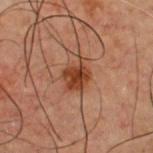The lesion was tiled from a total-body skin photograph and was not biopsied. On the chest. A close-up tile cropped from a whole-body skin photograph, about 15 mm across. Measured at roughly 3.5 mm in maximum diameter. A male subject aged around 60.